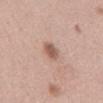The total-body-photography lesion software estimated a lesion area of about 4.5 mm², an outline eccentricity of about 0.85 (0 = round, 1 = elongated), and two-axis asymmetry of about 0.25. The analysis additionally found a lesion color around L≈57 a*≈19 b*≈26 in CIELAB, about 12 CIELAB-L* units darker than the surrounding skin, and a lesion-to-skin contrast of about 8 (normalized; higher = more distinct). The analysis additionally found a classifier nevus-likeness of about 95/100 and a detector confidence of about 100 out of 100 that the crop contains a lesion. A female subject aged approximately 60. Captured under white-light illumination. The lesion is located on the mid back. Approximately 3.5 mm at its widest. A 15 mm crop from a total-body photograph taken for skin-cancer surveillance.